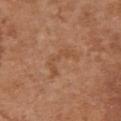{"biopsy_status": "not biopsied; imaged during a skin examination", "site": "chest", "image": {"source": "total-body photography crop", "field_of_view_mm": 15}, "patient": {"sex": "female", "age_approx": 65}}